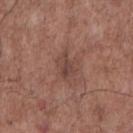biopsy status — catalogued during a skin exam; not biopsied | image source — ~15 mm crop, total-body skin-cancer survey | location — the chest | subject — male, roughly 70 years of age.